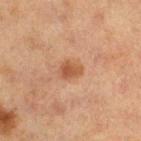This lesion was catalogued during total-body skin photography and was not selected for biopsy. The total-body-photography lesion software estimated a lesion area of about 4.5 mm², a shape eccentricity near 0.7, and a symmetry-axis asymmetry near 0.2. The software also gave a mean CIELAB color near L≈46 a*≈22 b*≈32, roughly 9 lightness units darker than nearby skin, and a lesion-to-skin contrast of about 7.5 (normalized; higher = more distinct). The software also gave a border-irregularity rating of about 2/10, a color-variation rating of about 4/10, and a peripheral color-asymmetry measure near 1.5. Located on the right thigh. A close-up tile cropped from a whole-body skin photograph, about 15 mm across. The lesion's longest dimension is about 2.5 mm. A female patient aged approximately 40. Imaged with cross-polarized lighting.